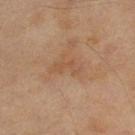Recorded during total-body skin imaging; not selected for excision or biopsy.